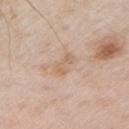Captured during whole-body skin photography for melanoma surveillance; the lesion was not biopsied.